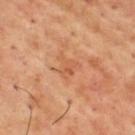biopsy status=imaged on a skin check; not biopsied | image source=~15 mm crop, total-body skin-cancer survey | body site=the back | illumination=cross-polarized | diameter=about 3 mm | patient=male, in their mid-50s.